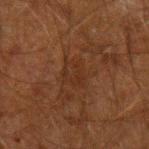| field | value |
|---|---|
| notes | no biopsy performed (imaged during a skin exam) |
| size | ≈3 mm |
| image | total-body-photography crop, ~15 mm field of view |
| anatomic site | the left forearm |
| lighting | cross-polarized |
| TBP lesion metrics | a lesion color around L≈23 a*≈17 b*≈24 in CIELAB and a normalized lesion–skin contrast near 4.5; border irregularity of about 3 on a 0–10 scale and peripheral color asymmetry of about 1; a classifier nevus-likeness of about 0/100 |
| subject | male, aged around 50 |